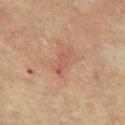{"lesion_size": {"long_diameter_mm_approx": 3.0}, "lighting": "cross-polarized", "site": "chest", "image": {"source": "total-body photography crop", "field_of_view_mm": 15}, "patient": {"sex": "male", "age_approx": 65}}